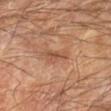<tbp_lesion>
  <biopsy_status>not biopsied; imaged during a skin examination</biopsy_status>
  <lighting>cross-polarized</lighting>
  <image>
    <source>total-body photography crop</source>
    <field_of_view_mm>15</field_of_view_mm>
  </image>
  <patient>
    <sex>male</sex>
    <age_approx>70</age_approx>
  </patient>
  <automated_metrics>
    <vs_skin_darker_L>8.0</vs_skin_darker_L>
    <vs_skin_contrast_norm>6.0</vs_skin_contrast_norm>
    <nevus_likeness_0_100>0</nevus_likeness_0_100>
    <lesion_detection_confidence_0_100>100</lesion_detection_confidence_0_100>
  </automated_metrics>
  <site>left forearm</site>
</tbp_lesion>